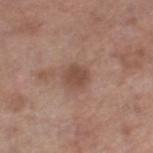Imaged during a routine full-body skin examination; the lesion was not biopsied and no histopathology is available. Captured under white-light illumination. The recorded lesion diameter is about 3 mm. The lesion is on the right thigh. A region of skin cropped from a whole-body photographic capture, roughly 15 mm wide. A male patient about 80 years old.